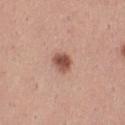This lesion was catalogued during total-body skin photography and was not selected for biopsy.
Captured under white-light illumination.
Measured at roughly 2.5 mm in maximum diameter.
The lesion is located on the left thigh.
Cropped from a total-body skin-imaging series; the visible field is about 15 mm.
A female patient aged around 30.